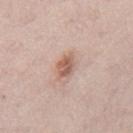biopsy status: total-body-photography surveillance lesion; no biopsy | site: the mid back | image-analysis metrics: an area of roughly 4.5 mm², a shape eccentricity near 0.7, and two-axis asymmetry of about 0.25; a mean CIELAB color near L≈59 a*≈20 b*≈27 and a normalized border contrast of about 8; a within-lesion color-variation index near 3/10 and radial color variation of about 1 | lighting: white-light | image source: ~15 mm crop, total-body skin-cancer survey | size: about 3 mm | patient: male, aged around 75.